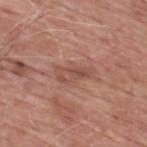Q: Lesion location?
A: the upper back
Q: How was the tile lit?
A: white-light
Q: How was this image acquired?
A: 15 mm crop, total-body photography
Q: What are the patient's age and sex?
A: male, aged approximately 60